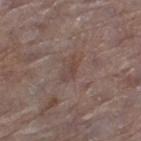notes = total-body-photography surveillance lesion; no biopsy | lesion size = ~3 mm (longest diameter) | imaging modality = ~15 mm crop, total-body skin-cancer survey | tile lighting = white-light illumination | body site = the left thigh | patient = female, aged around 85 or older.